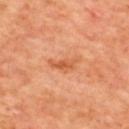biopsy status=total-body-photography surveillance lesion; no biopsy | automated lesion analysis=a footprint of about 4.5 mm², an eccentricity of roughly 0.9, and two-axis asymmetry of about 0.4; a border-irregularity index near 4.5/10 and radial color variation of about 1; a classifier nevus-likeness of about 0/100 | tile lighting=cross-polarized | patient=male, approximately 60 years of age | body site=the upper back | image=~15 mm crop, total-body skin-cancer survey.